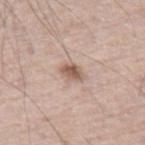Q: Was a biopsy performed?
A: imaged on a skin check; not biopsied
Q: Lesion location?
A: the left thigh
Q: How was the tile lit?
A: white-light illumination
Q: Automated lesion metrics?
A: a footprint of about 4 mm², an outline eccentricity of about 0.75 (0 = round, 1 = elongated), and a shape-asymmetry score of about 0.2 (0 = symmetric); a mean CIELAB color near L≈57 a*≈18 b*≈26, a lesion–skin lightness drop of about 13, and a normalized border contrast of about 8.5; border irregularity of about 2 on a 0–10 scale, internal color variation of about 3.5 on a 0–10 scale, and peripheral color asymmetry of about 1.5
Q: Who is the patient?
A: male, aged 68–72
Q: What kind of image is this?
A: ~15 mm tile from a whole-body skin photo
Q: How large is the lesion?
A: ≈2.5 mm